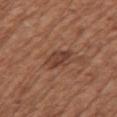workup = no biopsy performed (imaged during a skin exam) | location = the left upper arm | imaging modality = ~15 mm crop, total-body skin-cancer survey | subject = female, aged around 75.